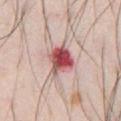The lesion was tiled from a total-body skin photograph and was not biopsied. The lesion's longest dimension is about 3.5 mm. This is a white-light tile. The lesion is on the chest. A male subject, aged 33–37. A roughly 15 mm field-of-view crop from a total-body skin photograph. Automated image analysis of the tile measured a footprint of about 9.5 mm² and an eccentricity of roughly 0.4. It also reported a lesion color around L≈54 a*≈30 b*≈22 in CIELAB and a normalized border contrast of about 11.5. And it measured a border-irregularity index near 2.5/10 and a peripheral color-asymmetry measure near 4.